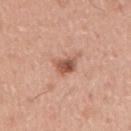Case summary:
* follow-up: catalogued during a skin exam; not biopsied
* size: ≈2.5 mm
* subject: male, in their mid- to late 40s
* automated metrics: an area of roughly 4.5 mm², a shape eccentricity near 0.55, and a symmetry-axis asymmetry near 0.25
* body site: the right upper arm
* tile lighting: white-light
* image source: ~15 mm crop, total-body skin-cancer survey